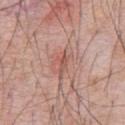{
  "biopsy_status": "not biopsied; imaged during a skin examination",
  "patient": {
    "sex": "male",
    "age_approx": 60
  },
  "site": "front of the torso",
  "automated_metrics": {
    "area_mm2_approx": 3.0,
    "eccentricity": 0.8,
    "border_irregularity_0_10": 4.5,
    "color_variation_0_10": 2.0,
    "peripheral_color_asymmetry": 1.0,
    "nevus_likeness_0_100": 25,
    "lesion_detection_confidence_0_100": 100
  },
  "image": {
    "source": "total-body photography crop",
    "field_of_view_mm": 15
  },
  "lesion_size": {
    "long_diameter_mm_approx": 2.5
  }
}